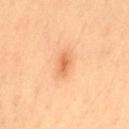follow-up: total-body-photography surveillance lesion; no biopsy | diameter: about 3 mm | automated metrics: an average lesion color of about L≈67 a*≈27 b*≈42 (CIELAB); internal color variation of about 2 on a 0–10 scale and radial color variation of about 0.5; a detector confidence of about 100 out of 100 that the crop contains a lesion | illumination: cross-polarized illumination | patient: female, in their 50s | site: the lower back | image source: ~15 mm crop, total-body skin-cancer survey.